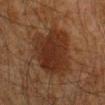Q: Was this lesion biopsied?
A: total-body-photography surveillance lesion; no biopsy
Q: Lesion location?
A: the right forearm
Q: Illumination type?
A: cross-polarized illumination
Q: What kind of image is this?
A: 15 mm crop, total-body photography
Q: Who is the patient?
A: male, in their mid-40s